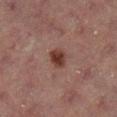{"biopsy_status": "not biopsied; imaged during a skin examination", "site": "right lower leg", "lesion_size": {"long_diameter_mm_approx": 2.5}, "image": {"source": "total-body photography crop", "field_of_view_mm": 15}, "patient": {"sex": "female", "age_approx": 70}}